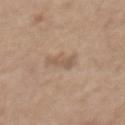Acquisition and patient details:
The lesion is located on the chest. The tile uses white-light illumination. Measured at roughly 3 mm in maximum diameter. A female patient, about 65 years old. This image is a 15 mm lesion crop taken from a total-body photograph.